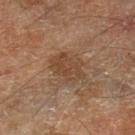follow-up: imaged on a skin check; not biopsied
lighting: cross-polarized
size: ~4.5 mm (longest diameter)
subject: male, roughly 70 years of age
image source: ~15 mm tile from a whole-body skin photo
image-analysis metrics: an area of roughly 9.5 mm², a shape eccentricity near 0.75, and a shape-asymmetry score of about 0.2 (0 = symmetric); an automated nevus-likeness rating near 5 out of 100 and a detector confidence of about 100 out of 100 that the crop contains a lesion
anatomic site: the leg